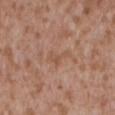Captured during whole-body skin photography for melanoma surveillance; the lesion was not biopsied. Located on the back. The subject is a male in their mid-40s. A 15 mm crop from a total-body photograph taken for skin-cancer surveillance.